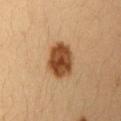The lesion was photographed on a routine skin check and not biopsied; there is no pathology result. A female patient, in their 30s. Captured under cross-polarized illumination. A close-up tile cropped from a whole-body skin photograph, about 15 mm across. The lesion is on the arm. Automated image analysis of the tile measured a lesion color around L≈42 a*≈20 b*≈34 in CIELAB and about 16 CIELAB-L* units darker than the surrounding skin. The software also gave border irregularity of about 2 on a 0–10 scale, a within-lesion color-variation index near 4.5/10, and a peripheral color-asymmetry measure near 1.5. It also reported an automated nevus-likeness rating near 100 out of 100. About 5 mm across.